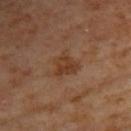Notes:
• biopsy status: catalogued during a skin exam; not biopsied
• image: total-body-photography crop, ~15 mm field of view
• site: the upper back
• TBP lesion metrics: a footprint of about 6 mm² and a shape-asymmetry score of about 0.45 (0 = symmetric); an average lesion color of about L≈36 a*≈20 b*≈30 (CIELAB) and about 7 CIELAB-L* units darker than the surrounding skin; a lesion-detection confidence of about 100/100
• subject: female, aged 63–67
• lighting: cross-polarized illumination